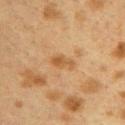Recorded during total-body skin imaging; not selected for excision or biopsy. Longest diameter approximately 3 mm. An algorithmic analysis of the crop reported an outline eccentricity of about 0.85 (0 = round, 1 = elongated) and two-axis asymmetry of about 0.25. The subject is a female aged approximately 40. Located on the upper back. The tile uses cross-polarized illumination. A 15 mm crop from a total-body photograph taken for skin-cancer surveillance.